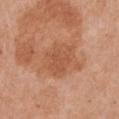follow-up: total-body-photography surveillance lesion; no biopsy
subject: female, approximately 55 years of age
acquisition: total-body-photography crop, ~15 mm field of view
body site: the front of the torso
illumination: white-light illumination
lesion diameter: ~4.5 mm (longest diameter)